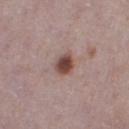The lesion was tiled from a total-body skin photograph and was not biopsied. The patient is a female aged around 30. A lesion tile, about 15 mm wide, cut from a 3D total-body photograph. An algorithmic analysis of the crop reported a mean CIELAB color near L≈46 a*≈20 b*≈22 and a normalized lesion–skin contrast near 10.5. Measured at roughly 2.5 mm in maximum diameter. The tile uses white-light illumination. Located on the right thigh.